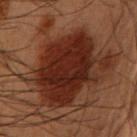No biopsy was performed on this lesion — it was imaged during a full skin examination and was not determined to be concerning.
A lesion tile, about 15 mm wide, cut from a 3D total-body photograph.
The subject is a male aged around 55.
This is a cross-polarized tile.
The lesion is located on the arm.